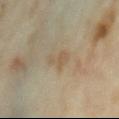Imaged during a routine full-body skin examination; the lesion was not biopsied and no histopathology is available. Measured at roughly 3.5 mm in maximum diameter. Located on the chest. This is a cross-polarized tile. The subject is a female aged 33 to 37. A 15 mm close-up extracted from a 3D total-body photography capture. The total-body-photography lesion software estimated an eccentricity of roughly 0.9 and a shape-asymmetry score of about 0.45 (0 = symmetric). And it measured internal color variation of about 1 on a 0–10 scale and peripheral color asymmetry of about 0.5.